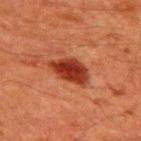<record>
<biopsy_status>not biopsied; imaged during a skin examination</biopsy_status>
<lighting>cross-polarized</lighting>
<image>
  <source>total-body photography crop</source>
  <field_of_view_mm>15</field_of_view_mm>
</image>
<lesion_size>
  <long_diameter_mm_approx>5.0</long_diameter_mm_approx>
</lesion_size>
<automated_metrics>
  <eccentricity>0.85</eccentricity>
  <shape_asymmetry>0.3</shape_asymmetry>
  <border_irregularity_0_10>3.0</border_irregularity_0_10>
  <color_variation_0_10>3.5</color_variation_0_10>
  <peripheral_color_asymmetry>1.0</peripheral_color_asymmetry>
</automated_metrics>
<patient>
  <sex>male</sex>
  <age_approx>60</age_approx>
</patient>
<site>upper back</site>
</record>